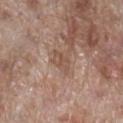notes: total-body-photography surveillance lesion; no biopsy | image-analysis metrics: a footprint of about 5 mm² and a shape-asymmetry score of about 0.25 (0 = symmetric) | image: 15 mm crop, total-body photography | tile lighting: white-light | site: the right lower leg | patient: male, aged 68 to 72 | lesion diameter: ≈3 mm.